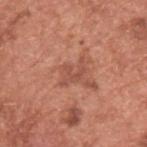The patient is a male aged around 65.
On the upper back.
Captured under white-light illumination.
A region of skin cropped from a whole-body photographic capture, roughly 15 mm wide.
The recorded lesion diameter is about 3.5 mm.
Automated image analysis of the tile measured an outline eccentricity of about 0.8 (0 = round, 1 = elongated) and a shape-asymmetry score of about 0.5 (0 = symmetric). It also reported a border-irregularity rating of about 7.5/10, a color-variation rating of about 0/10, and a peripheral color-asymmetry measure near 0. The analysis additionally found a classifier nevus-likeness of about 0/100 and a detector confidence of about 100 out of 100 that the crop contains a lesion.